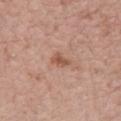Q: Patient demographics?
A: male, about 50 years old
Q: How was this image acquired?
A: 15 mm crop, total-body photography
Q: Where on the body is the lesion?
A: the back
Q: What lighting was used for the tile?
A: white-light illumination